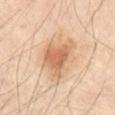Case summary:
• workup · no biopsy performed (imaged during a skin exam)
• image · ~15 mm tile from a whole-body skin photo
• TBP lesion metrics · an area of roughly 14 mm², an outline eccentricity of about 0.7 (0 = round, 1 = elongated), and two-axis asymmetry of about 0.45; a border-irregularity rating of about 4.5/10 and peripheral color asymmetry of about 1
• site · the abdomen